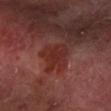Imaged during a routine full-body skin examination; the lesion was not biopsied and no histopathology is available.
The lesion's longest dimension is about 3.5 mm.
The lesion is on the left forearm.
Automated tile analysis of the lesion measured a lesion color around L≈28 a*≈26 b*≈23 in CIELAB and roughly 6 lightness units darker than nearby skin. The software also gave a within-lesion color-variation index near 3/10 and peripheral color asymmetry of about 1.
A close-up tile cropped from a whole-body skin photograph, about 15 mm across.
A male subject about 65 years old.